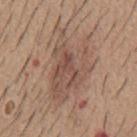The patient is a male aged around 60. The lesion is on the mid back. This is a white-light tile. A 15 mm crop from a total-body photograph taken for skin-cancer surveillance. The lesion's longest dimension is about 8.5 mm.